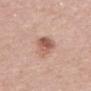Captured during whole-body skin photography for melanoma surveillance; the lesion was not biopsied.
Located on the back.
A 15 mm close-up tile from a total-body photography series done for melanoma screening.
Longest diameter approximately 4.5 mm.
The lesion-visualizer software estimated a mean CIELAB color near L≈57 a*≈23 b*≈27 and a lesion–skin lightness drop of about 12. The analysis additionally found an automated nevus-likeness rating near 70 out of 100 and a lesion-detection confidence of about 100/100.
Captured under white-light illumination.
A female patient about 65 years old.